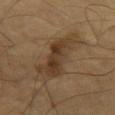Captured during whole-body skin photography for melanoma surveillance; the lesion was not biopsied.
A male patient, about 60 years old.
The lesion's longest dimension is about 7 mm.
Located on the chest.
Automated image analysis of the tile measured a border-irregularity rating of about 4/10, internal color variation of about 4.5 on a 0–10 scale, and radial color variation of about 1.5.
A 15 mm crop from a total-body photograph taken for skin-cancer surveillance.